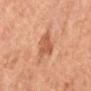| feature | finding |
|---|---|
| follow-up | total-body-photography surveillance lesion; no biopsy |
| image-analysis metrics | a footprint of about 7 mm² and an outline eccentricity of about 0.75 (0 = round, 1 = elongated); an automated nevus-likeness rating near 10 out of 100 and lesion-presence confidence of about 100/100 |
| body site | the right forearm |
| imaging modality | ~15 mm tile from a whole-body skin photo |
| patient | female, aged approximately 65 |